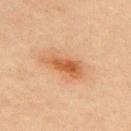  biopsy_status: not biopsied; imaged during a skin examination
  lighting: cross-polarized
  lesion_size:
    long_diameter_mm_approx: 5.5
  patient:
    sex: female
    age_approx: 45
  site: upper back
  image:
    source: total-body photography crop
    field_of_view_mm: 15